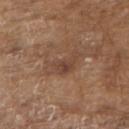Recorded during total-body skin imaging; not selected for excision or biopsy.
A lesion tile, about 15 mm wide, cut from a 3D total-body photograph.
A male subject, aged 78–82.
Located on the upper back.
The lesion's longest dimension is about 5 mm.